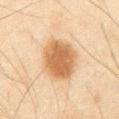workup = catalogued during a skin exam; not biopsied | location = the abdomen | imaging modality = 15 mm crop, total-body photography | patient = male, approximately 65 years of age.